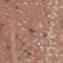Part of a total-body skin-imaging series; this lesion was reviewed on a skin check and was not flagged for biopsy. A 15 mm crop from a total-body photograph taken for skin-cancer surveillance. About 3.5 mm across. A male patient, aged around 55. On the chest.